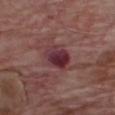Case summary:
• follow-up: imaged on a skin check; not biopsied
• patient: male, aged 63–67
• anatomic site: the chest
• image source: total-body-photography crop, ~15 mm field of view